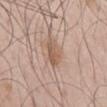The lesion was tiled from a total-body skin photograph and was not biopsied.
Located on the mid back.
Cropped from a total-body skin-imaging series; the visible field is about 15 mm.
The patient is a male approximately 45 years of age.